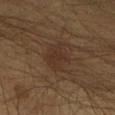Clinical impression:
The lesion was tiled from a total-body skin photograph and was not biopsied.
Acquisition and patient details:
The recorded lesion diameter is about 4 mm. Automated tile analysis of the lesion measured a lesion area of about 9 mm², an outline eccentricity of about 0.7 (0 = round, 1 = elongated), and a shape-asymmetry score of about 0.25 (0 = symmetric). The software also gave a mean CIELAB color near L≈27 a*≈14 b*≈22, about 5 CIELAB-L* units darker than the surrounding skin, and a lesion-to-skin contrast of about 5.5 (normalized; higher = more distinct). The analysis additionally found border irregularity of about 2.5 on a 0–10 scale, internal color variation of about 2 on a 0–10 scale, and a peripheral color-asymmetry measure near 0.5. This is a cross-polarized tile. The subject is a male aged approximately 65. The lesion is on the front of the torso. A 15 mm close-up extracted from a 3D total-body photography capture.